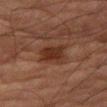The lesion was photographed on a routine skin check and not biopsied; there is no pathology result. This is a cross-polarized tile. Cropped from a whole-body photographic skin survey; the tile spans about 15 mm. The lesion is located on the right thigh. A male patient, roughly 80 years of age.